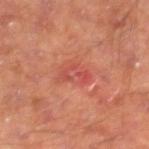Clinical impression:
The lesion was photographed on a routine skin check and not biopsied; there is no pathology result.
Background:
This is a cross-polarized tile. Located on the right lower leg. A male subject, in their mid- to late 60s. The lesion's longest dimension is about 3.5 mm. A 15 mm close-up extracted from a 3D total-body photography capture.